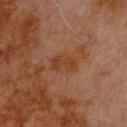Clinical impression: No biopsy was performed on this lesion — it was imaged during a full skin examination and was not determined to be concerning. Background: The lesion is on the upper back. Captured under cross-polarized illumination. A male patient, aged 78 to 82. A lesion tile, about 15 mm wide, cut from a 3D total-body photograph. About 3 mm across.